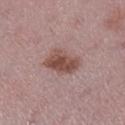This lesion was catalogued during total-body skin photography and was not selected for biopsy. The lesion is on the leg. Automated tile analysis of the lesion measured a lesion area of about 9.5 mm², an outline eccentricity of about 0.75 (0 = round, 1 = elongated), and two-axis asymmetry of about 0.3. The analysis additionally found an automated nevus-likeness rating near 70 out of 100 and lesion-presence confidence of about 100/100. The patient is a female in their mid-40s. A 15 mm crop from a total-body photograph taken for skin-cancer surveillance. The recorded lesion diameter is about 4 mm.